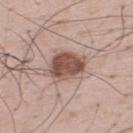{
  "biopsy_status": "not biopsied; imaged during a skin examination"
}